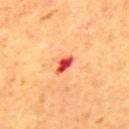workup = total-body-photography surveillance lesion; no biopsy | lesion size = ≈2.5 mm | automated lesion analysis = a footprint of about 3 mm² and a shape eccentricity near 0.85; about 19 CIELAB-L* units darker than the surrounding skin; a border-irregularity index near 3/10, internal color variation of about 2.5 on a 0–10 scale, and peripheral color asymmetry of about 0.5; a classifier nevus-likeness of about 0/100 and a lesion-detection confidence of about 100/100 | subject = male, approximately 55 years of age | anatomic site = the mid back | image source = 15 mm crop, total-body photography | lighting = cross-polarized illumination.